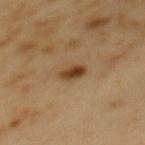Impression:
No biopsy was performed on this lesion — it was imaged during a full skin examination and was not determined to be concerning.
Clinical summary:
A female subject aged 48–52. Captured under cross-polarized illumination. The recorded lesion diameter is about 2.5 mm. A close-up tile cropped from a whole-body skin photograph, about 15 mm across. On the mid back.